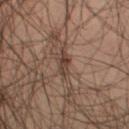| feature | finding |
|---|---|
| workup | no biopsy performed (imaged during a skin exam) |
| subject | male, roughly 50 years of age |
| illumination | cross-polarized |
| diameter | about 2.5 mm |
| image | ~15 mm crop, total-body skin-cancer survey |
| site | the right thigh |